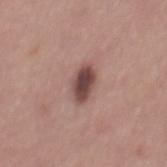Part of a total-body skin-imaging series; this lesion was reviewed on a skin check and was not flagged for biopsy. The subject is a male approximately 40 years of age. From the mid back. Approximately 4 mm at its widest. The tile uses white-light illumination. A roughly 15 mm field-of-view crop from a total-body skin photograph.